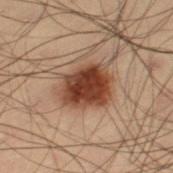notes: catalogued during a skin exam; not biopsied | image-analysis metrics: a footprint of about 17 mm², an outline eccentricity of about 0.6 (0 = round, 1 = elongated), and two-axis asymmetry of about 0.2; border irregularity of about 2.5 on a 0–10 scale, a within-lesion color-variation index near 5/10, and peripheral color asymmetry of about 1; a nevus-likeness score of about 100/100 | body site: the leg | patient: male, aged approximately 55 | imaging modality: total-body-photography crop, ~15 mm field of view | size: about 5 mm | lighting: cross-polarized.